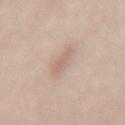Clinical impression:
The lesion was photographed on a routine skin check and not biopsied; there is no pathology result.
Image and clinical context:
A female patient, aged approximately 75. The tile uses white-light illumination. The lesion-visualizer software estimated an area of roughly 5.5 mm², a shape eccentricity near 0.85, and a shape-asymmetry score of about 0.25 (0 = symmetric). It also reported an automated nevus-likeness rating near 0 out of 100 and a detector confidence of about 100 out of 100 that the crop contains a lesion. A roughly 15 mm field-of-view crop from a total-body skin photograph. The recorded lesion diameter is about 4 mm. Located on the mid back.